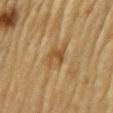Impression:
The lesion was tiled from a total-body skin photograph and was not biopsied.
Context:
The lesion-visualizer software estimated a lesion area of about 6 mm², an outline eccentricity of about 0.75 (0 = round, 1 = elongated), and a symmetry-axis asymmetry near 0.3. The software also gave border irregularity of about 3.5 on a 0–10 scale, internal color variation of about 4 on a 0–10 scale, and peripheral color asymmetry of about 1. And it measured a nevus-likeness score of about 5/100. A male subject, roughly 85 years of age or older. This image is a 15 mm lesion crop taken from a total-body photograph. Approximately 3.5 mm at its widest. Located on the left upper arm.